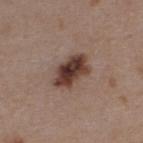Q: Is there a histopathology result?
A: total-body-photography surveillance lesion; no biopsy
Q: Lesion location?
A: the upper back
Q: What lighting was used for the tile?
A: white-light illumination
Q: Lesion size?
A: ~4.5 mm (longest diameter)
Q: How was this image acquired?
A: total-body-photography crop, ~15 mm field of view
Q: What are the patient's age and sex?
A: female, aged around 45